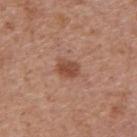Clinical impression: Recorded during total-body skin imaging; not selected for excision or biopsy. Background: Imaged with white-light lighting. On the upper back. The subject is a male approximately 70 years of age. Measured at roughly 2.5 mm in maximum diameter. Automated image analysis of the tile measured a shape eccentricity near 0.7. The software also gave an average lesion color of about L≈47 a*≈24 b*≈31 (CIELAB), roughly 10 lightness units darker than nearby skin, and a normalized lesion–skin contrast near 8. The software also gave a lesion-detection confidence of about 100/100. A region of skin cropped from a whole-body photographic capture, roughly 15 mm wide.